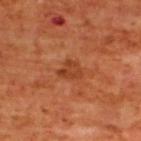Imaged during a routine full-body skin examination; the lesion was not biopsied and no histopathology is available.